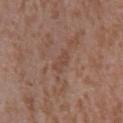• notes: imaged on a skin check; not biopsied
• patient: male, in their mid-40s
• TBP lesion metrics: border irregularity of about 4 on a 0–10 scale, internal color variation of about 0 on a 0–10 scale, and a peripheral color-asymmetry measure near 0; a nevus-likeness score of about 0/100 and lesion-presence confidence of about 60/100
• site: the chest
• imaging modality: total-body-photography crop, ~15 mm field of view